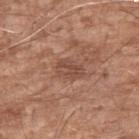Notes:
* follow-up: no biopsy performed (imaged during a skin exam)
* lesion size: about 3 mm
* illumination: white-light illumination
* site: the left upper arm
* acquisition: 15 mm crop, total-body photography
* patient: male, roughly 65 years of age